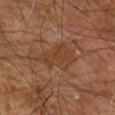Imaged during a routine full-body skin examination; the lesion was not biopsied and no histopathology is available.
The patient is a male aged around 60.
A 15 mm close-up extracted from a 3D total-body photography capture.
The lesion is located on the right leg.
The lesion-visualizer software estimated a footprint of about 11 mm² and an outline eccentricity of about 0.75 (0 = round, 1 = elongated). And it measured border irregularity of about 4.5 on a 0–10 scale.